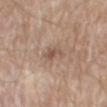  biopsy_status: not biopsied; imaged during a skin examination
  patient:
    sex: male
    age_approx: 80
  lighting: white-light
  lesion_size:
    long_diameter_mm_approx: 3.0
  site: mid back
  image:
    source: total-body photography crop
    field_of_view_mm: 15